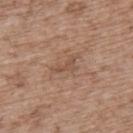{
  "biopsy_status": "not biopsied; imaged during a skin examination",
  "image": {
    "source": "total-body photography crop",
    "field_of_view_mm": 15
  },
  "site": "upper back",
  "patient": {
    "sex": "male",
    "age_approx": 70
  },
  "lighting": "white-light",
  "lesion_size": {
    "long_diameter_mm_approx": 3.0
  },
  "automated_metrics": {
    "cielab_L": 51,
    "cielab_a": 18,
    "cielab_b": 28,
    "vs_skin_darker_L": 7.0,
    "border_irregularity_0_10": 5.0,
    "color_variation_0_10": 0.0,
    "peripheral_color_asymmetry": 0.0,
    "nevus_likeness_0_100": 0,
    "lesion_detection_confidence_0_100": 100
  }
}